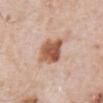<case>
<biopsy_status>not biopsied; imaged during a skin examination</biopsy_status>
<lesion_size>
  <long_diameter_mm_approx>3.5</long_diameter_mm_approx>
</lesion_size>
<site>abdomen</site>
<image>
  <source>total-body photography crop</source>
  <field_of_view_mm>15</field_of_view_mm>
</image>
<automated_metrics>
  <border_irregularity_0_10>2.0</border_irregularity_0_10>
  <color_variation_0_10>6.0</color_variation_0_10>
</automated_metrics>
<patient>
  <sex>male</sex>
  <age_approx>80</age_approx>
</patient>
</case>